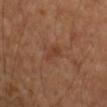follow-up: total-body-photography surveillance lesion; no biopsy
location: the right upper arm
illumination: cross-polarized
subject: female, about 60 years old
image-analysis metrics: a footprint of about 3 mm², an eccentricity of roughly 0.85, and a shape-asymmetry score of about 0.3 (0 = symmetric); a border-irregularity index near 2.5/10 and radial color variation of about 0.5; a nevus-likeness score of about 5/100 and lesion-presence confidence of about 100/100
image source: total-body-photography crop, ~15 mm field of view
lesion diameter: about 2.5 mm